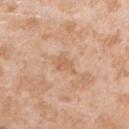subject: female, in their mid- to late 20s
site: the arm
acquisition: total-body-photography crop, ~15 mm field of view
lesion diameter: about 3 mm